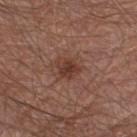No biopsy was performed on this lesion — it was imaged during a full skin examination and was not determined to be concerning.
A lesion tile, about 15 mm wide, cut from a 3D total-body photograph.
This is a white-light tile.
An algorithmic analysis of the crop reported a mean CIELAB color near L≈38 a*≈21 b*≈25 and a normalized lesion–skin contrast near 7.5. The analysis additionally found a border-irregularity rating of about 2.5/10, a color-variation rating of about 4.5/10, and peripheral color asymmetry of about 1.5. It also reported a nevus-likeness score of about 80/100 and lesion-presence confidence of about 100/100.
The lesion is located on the leg.
Approximately 2.5 mm at its widest.
A male patient, aged 63 to 67.